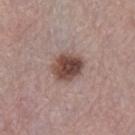This lesion was catalogued during total-body skin photography and was not selected for biopsy. The lesion's longest dimension is about 4 mm. On the leg. A female patient approximately 65 years of age. A close-up tile cropped from a whole-body skin photograph, about 15 mm across. Imaged with white-light lighting.